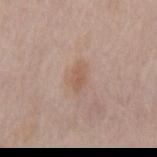Impression:
The lesion was tiled from a total-body skin photograph and was not biopsied.
Acquisition and patient details:
The total-body-photography lesion software estimated an area of roughly 3.5 mm², an eccentricity of roughly 0.85, and a shape-asymmetry score of about 0.2 (0 = symmetric). The analysis additionally found an average lesion color of about L≈56 a*≈18 b*≈27 (CIELAB), a lesion–skin lightness drop of about 7, and a normalized lesion–skin contrast near 6. And it measured a nevus-likeness score of about 55/100. This is a white-light tile. Longest diameter approximately 3 mm. On the mid back. A male patient, in their mid-70s. A lesion tile, about 15 mm wide, cut from a 3D total-body photograph.